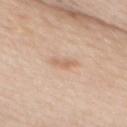| field | value |
|---|---|
| workup | total-body-photography surveillance lesion; no biopsy |
| automated metrics | an area of roughly 3 mm² and a shape eccentricity near 0.95; a mean CIELAB color near L≈64 a*≈19 b*≈32 and about 8 CIELAB-L* units darker than the surrounding skin; a border-irregularity index near 4.5/10, a color-variation rating of about 0/10, and radial color variation of about 0; a classifier nevus-likeness of about 10/100 and a detector confidence of about 100 out of 100 that the crop contains a lesion |
| patient | male, aged 58 to 62 |
| lesion size | about 3 mm |
| tile lighting | white-light |
| image source | total-body-photography crop, ~15 mm field of view |
| body site | the back |